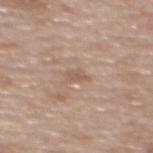| field | value |
|---|---|
| follow-up | catalogued during a skin exam; not biopsied |
| acquisition | ~15 mm tile from a whole-body skin photo |
| patient | female, aged 58–62 |
| lesion size | ~3 mm (longest diameter) |
| anatomic site | the upper back |